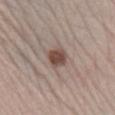The lesion was photographed on a routine skin check and not biopsied; there is no pathology result. Imaged with white-light lighting. From the right lower leg. A male patient aged 63 to 67. The lesion-visualizer software estimated an eccentricity of roughly 0.3. And it measured a mean CIELAB color near L≈47 a*≈17 b*≈23, about 13 CIELAB-L* units darker than the surrounding skin, and a lesion-to-skin contrast of about 10 (normalized; higher = more distinct). The software also gave a border-irregularity index near 1.5/10, a within-lesion color-variation index near 2/10, and a peripheral color-asymmetry measure near 0.5. About 2.5 mm across. A lesion tile, about 15 mm wide, cut from a 3D total-body photograph.